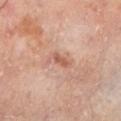Q: Was this lesion biopsied?
A: catalogued during a skin exam; not biopsied
Q: Automated lesion metrics?
A: a footprint of about 3 mm², an outline eccentricity of about 0.9 (0 = round, 1 = elongated), and a shape-asymmetry score of about 0.35 (0 = symmetric); a lesion color around L≈55 a*≈22 b*≈29 in CIELAB, a lesion–skin lightness drop of about 9, and a normalized lesion–skin contrast near 6.5; a border-irregularity index near 3.5/10, a within-lesion color-variation index near 0/10, and peripheral color asymmetry of about 0; a detector confidence of about 100 out of 100 that the crop contains a lesion
Q: What kind of image is this?
A: 15 mm crop, total-body photography
Q: How was the tile lit?
A: cross-polarized illumination
Q: Where on the body is the lesion?
A: the left lower leg
Q: What are the patient's age and sex?
A: male, in their mid-60s